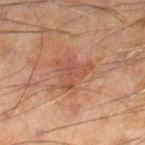No biopsy was performed on this lesion — it was imaged during a full skin examination and was not determined to be concerning.
Automated tile analysis of the lesion measured a lesion area of about 9 mm², an outline eccentricity of about 0.45 (0 = round, 1 = elongated), and two-axis asymmetry of about 0.55. The software also gave an average lesion color of about L≈38 a*≈19 b*≈24 (CIELAB) and a normalized border contrast of about 5.5. And it measured internal color variation of about 2 on a 0–10 scale. The analysis additionally found a lesion-detection confidence of about 100/100.
The subject is a male approximately 60 years of age.
A region of skin cropped from a whole-body photographic capture, roughly 15 mm wide.
Located on the left leg.
Imaged with cross-polarized lighting.
Measured at roughly 4 mm in maximum diameter.